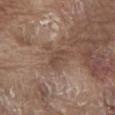Assessment:
The lesion was photographed on a routine skin check and not biopsied; there is no pathology result.
Image and clinical context:
Imaged with white-light lighting. Cropped from a total-body skin-imaging series; the visible field is about 15 mm. Located on the abdomen. Measured at roughly 3 mm in maximum diameter. A male subject about 80 years old. Automated image analysis of the tile measured border irregularity of about 4.5 on a 0–10 scale, a within-lesion color-variation index near 1.5/10, and a peripheral color-asymmetry measure near 0.5. The analysis additionally found a nevus-likeness score of about 0/100 and a detector confidence of about 100 out of 100 that the crop contains a lesion.